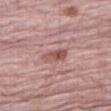biopsy status: catalogued during a skin exam; not biopsied | acquisition: total-body-photography crop, ~15 mm field of view | illumination: white-light illumination | site: the right thigh | subject: male, approximately 70 years of age | lesion size: ~3 mm (longest diameter) | TBP lesion metrics: an average lesion color of about L≈53 a*≈23 b*≈23 (CIELAB) and a lesion–skin lightness drop of about 10; a border-irregularity index near 3.5/10 and a color-variation rating of about 6/10; a classifier nevus-likeness of about 30/100.